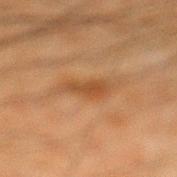Clinical impression: The lesion was tiled from a total-body skin photograph and was not biopsied.